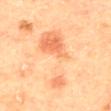biopsy status=catalogued during a skin exam; not biopsied | patient=female, about 60 years old | body site=the mid back | imaging modality=~15 mm crop, total-body skin-cancer survey | lesion diameter=≈8 mm | automated lesion analysis=a lesion area of about 23 mm² and an eccentricity of roughly 0.9; an average lesion color of about L≈70 a*≈26 b*≈40 (CIELAB) | illumination=cross-polarized illumination.